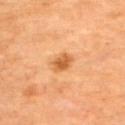Q: Is there a histopathology result?
A: catalogued during a skin exam; not biopsied
Q: Illumination type?
A: cross-polarized illumination
Q: What is the lesion's diameter?
A: ≈2.5 mm
Q: Automated lesion metrics?
A: a lesion area of about 5 mm², an eccentricity of roughly 0.55, and a symmetry-axis asymmetry near 0.2; a peripheral color-asymmetry measure near 1; a classifier nevus-likeness of about 75/100 and a detector confidence of about 100 out of 100 that the crop contains a lesion
Q: What is the imaging modality?
A: ~15 mm crop, total-body skin-cancer survey
Q: Lesion location?
A: the upper back
Q: Patient demographics?
A: female, roughly 70 years of age